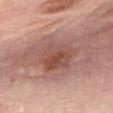Case summary:
• size: about 6 mm
• subject: female, aged approximately 60
• image source: total-body-photography crop, ~15 mm field of view
• anatomic site: the front of the torso
• lighting: white-light illumination